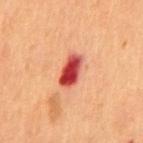biopsy_status: not biopsied; imaged during a skin examination
image:
  source: total-body photography crop
  field_of_view_mm: 15
patient:
  sex: male
  age_approx: 55
site: abdomen